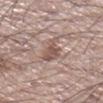The lesion was tiled from a total-body skin photograph and was not biopsied.
Approximately 4 mm at its widest.
The lesion-visualizer software estimated a lesion area of about 7 mm² and an outline eccentricity of about 0.8 (0 = round, 1 = elongated). And it measured a border-irregularity rating of about 4.5/10 and radial color variation of about 1.
This is a white-light tile.
A male patient, aged around 60.
A 15 mm close-up extracted from a 3D total-body photography capture.
The lesion is located on the right lower leg.